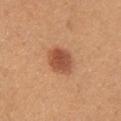tile lighting = white-light
diameter = about 3.5 mm
site = the upper back
subject = female, in their mid- to late 20s
image = ~15 mm crop, total-body skin-cancer survey